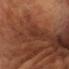<lesion>
  <biopsy_status>not biopsied; imaged during a skin examination</biopsy_status>
  <patient>
    <sex>male</sex>
    <age_approx>65</age_approx>
  </patient>
  <lesion_size>
    <long_diameter_mm_approx>3.0</long_diameter_mm_approx>
  </lesion_size>
  <lighting>cross-polarized</lighting>
  <image>
    <source>total-body photography crop</source>
    <field_of_view_mm>15</field_of_view_mm>
  </image>
  <site>head or neck</site>
</lesion>